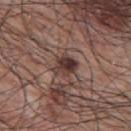The lesion was photographed on a routine skin check and not biopsied; there is no pathology result.
A male subject roughly 70 years of age.
Measured at roughly 3 mm in maximum diameter.
On the upper back.
A lesion tile, about 15 mm wide, cut from a 3D total-body photograph.